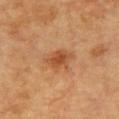Impression: Imaged during a routine full-body skin examination; the lesion was not biopsied and no histopathology is available. Image and clinical context: This image is a 15 mm lesion crop taken from a total-body photograph. Approximately 3.5 mm at its widest. A male subject, approximately 85 years of age or older. The tile uses cross-polarized illumination. The total-body-photography lesion software estimated a mean CIELAB color near L≈44 a*≈22 b*≈34, roughly 9 lightness units darker than nearby skin, and a lesion-to-skin contrast of about 7 (normalized; higher = more distinct). The software also gave a border-irregularity index near 2/10, a within-lesion color-variation index near 3.5/10, and radial color variation of about 1. The software also gave a classifier nevus-likeness of about 65/100 and lesion-presence confidence of about 100/100. The lesion is on the chest.